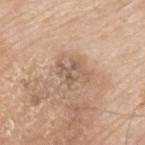The lesion's longest dimension is about 3.5 mm.
This is a white-light tile.
An algorithmic analysis of the crop reported a border-irregularity rating of about 6.5/10, internal color variation of about 4.5 on a 0–10 scale, and radial color variation of about 1.5.
A male patient approximately 80 years of age.
A 15 mm close-up tile from a total-body photography series done for melanoma screening.
The lesion is located on the upper back.